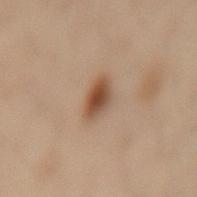The lesion was photographed on a routine skin check and not biopsied; there is no pathology result.
This is a cross-polarized tile.
A female patient, aged around 55.
Approximately 3.5 mm at its widest.
A 15 mm crop from a total-body photograph taken for skin-cancer surveillance.
The lesion is located on the mid back.